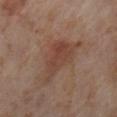notes: imaged on a skin check; not biopsied | location: the right lower leg | image: 15 mm crop, total-body photography | subject: female, in their mid-50s | image-analysis metrics: an eccentricity of roughly 0.75 and two-axis asymmetry of about 0.45; a lesion–skin lightness drop of about 7 and a normalized border contrast of about 6; border irregularity of about 5 on a 0–10 scale, a color-variation rating of about 4/10, and a peripheral color-asymmetry measure near 1.5.